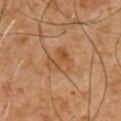This is a cross-polarized tile. The recorded lesion diameter is about 3.5 mm. A male patient aged around 60. On the front of the torso. Cropped from a whole-body photographic skin survey; the tile spans about 15 mm.